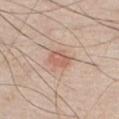<case>
<biopsy_status>not biopsied; imaged during a skin examination</biopsy_status>
<lighting>white-light</lighting>
<site>chest</site>
<lesion_size>
  <long_diameter_mm_approx>3.0</long_diameter_mm_approx>
</lesion_size>
<patient>
  <sex>male</sex>
  <age_approx>45</age_approx>
</patient>
<automated_metrics>
  <cielab_L>60</cielab_L>
  <cielab_a>22</cielab_a>
  <cielab_b>28</cielab_b>
</automated_metrics>
<image>
  <source>total-body photography crop</source>
  <field_of_view_mm>15</field_of_view_mm>
</image>
</case>